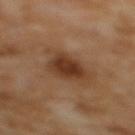Imaged during a routine full-body skin examination; the lesion was not biopsied and no histopathology is available. On the mid back. A female subject roughly 55 years of age. Longest diameter approximately 4 mm. A 15 mm crop from a total-body photograph taken for skin-cancer surveillance.